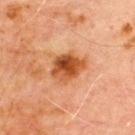Findings:
* biopsy status — total-body-photography surveillance lesion; no biopsy
* anatomic site — the front of the torso
* diameter — about 4 mm
* tile lighting — cross-polarized
* patient — male, aged around 70
* TBP lesion metrics — an outline eccentricity of about 0.5 (0 = round, 1 = elongated) and a shape-asymmetry score of about 0.2 (0 = symmetric); a lesion–skin lightness drop of about 13 and a normalized lesion–skin contrast near 10; an automated nevus-likeness rating near 95 out of 100
* image — ~15 mm crop, total-body skin-cancer survey